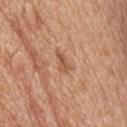Cropped from a whole-body photographic skin survey; the tile spans about 15 mm. The lesion-visualizer software estimated a lesion area of about 3 mm² and a shape-asymmetry score of about 0.3 (0 = symmetric). The software also gave a lesion color around L≈55 a*≈22 b*≈33 in CIELAB, roughly 9 lightness units darker than nearby skin, and a normalized border contrast of about 6.5. And it measured a border-irregularity index near 3.5/10. Located on the head or neck. A male patient, in their 80s. Captured under white-light illumination.